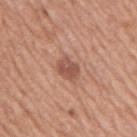Q: Was this lesion biopsied?
A: catalogued during a skin exam; not biopsied
Q: Illumination type?
A: white-light illumination
Q: What is the imaging modality?
A: ~15 mm crop, total-body skin-cancer survey
Q: Lesion location?
A: the right upper arm
Q: What is the lesion's diameter?
A: about 3 mm
Q: Who is the patient?
A: male, aged 73–77